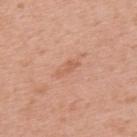Notes:
• notes — total-body-photography surveillance lesion; no biopsy
• patient — male, aged 58–62
• image source — ~15 mm crop, total-body skin-cancer survey
• automated lesion analysis — an eccentricity of roughly 0.9 and a symmetry-axis asymmetry near 0.35; a lesion color around L≈60 a*≈24 b*≈33 in CIELAB and roughly 7 lightness units darker than nearby skin; an automated nevus-likeness rating near 0 out of 100 and a lesion-detection confidence of about 100/100
• tile lighting — white-light illumination
• anatomic site — the upper back